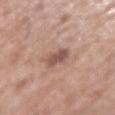Q: Is there a histopathology result?
A: catalogued during a skin exam; not biopsied
Q: Who is the patient?
A: male, aged 73–77
Q: How large is the lesion?
A: ≈2.5 mm
Q: Where on the body is the lesion?
A: the left upper arm
Q: What kind of image is this?
A: 15 mm crop, total-body photography
Q: What did automated image analysis measure?
A: a lesion area of about 5 mm², an eccentricity of roughly 0.15, and a symmetry-axis asymmetry near 0.2; an automated nevus-likeness rating near 40 out of 100 and a detector confidence of about 100 out of 100 that the crop contains a lesion
Q: What lighting was used for the tile?
A: white-light illumination